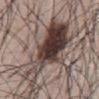Notes:
* notes: no biopsy performed (imaged during a skin exam)
* image-analysis metrics: a footprint of about 32 mm² and an outline eccentricity of about 0.85 (0 = round, 1 = elongated); a lesion color around L≈40 a*≈13 b*≈19 in CIELAB, about 18 CIELAB-L* units darker than the surrounding skin, and a lesion-to-skin contrast of about 13.5 (normalized; higher = more distinct); a peripheral color-asymmetry measure near 4.5; a nevus-likeness score of about 100/100 and a lesion-detection confidence of about 100/100
* size: about 10.5 mm
* imaging modality: ~15 mm crop, total-body skin-cancer survey
* illumination: white-light illumination
* site: the abdomen
* patient: male, approximately 70 years of age